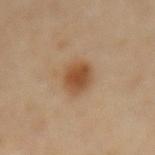Context: The patient is a female aged 48–52. The lesion is located on the lower back. Imaged with cross-polarized lighting. The lesion's longest dimension is about 3.5 mm. A 15 mm crop from a total-body photograph taken for skin-cancer surveillance. The lesion-visualizer software estimated an area of roughly 8.5 mm², a shape eccentricity near 0.65, and two-axis asymmetry of about 0.1. It also reported a nevus-likeness score of about 100/100.